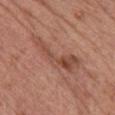The lesion was tiled from a total-body skin photograph and was not biopsied. Imaged with white-light lighting. Cropped from a total-body skin-imaging series; the visible field is about 15 mm. On the chest. Automated image analysis of the tile measured a footprint of about 12 mm², an eccentricity of roughly 0.95, and a shape-asymmetry score of about 0.55 (0 = symmetric). The software also gave internal color variation of about 6 on a 0–10 scale and peripheral color asymmetry of about 2. The analysis additionally found a lesion-detection confidence of about 100/100. A female subject, aged approximately 60. Measured at roughly 7.5 mm in maximum diameter.